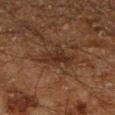Recorded during total-body skin imaging; not selected for excision or biopsy.
A region of skin cropped from a whole-body photographic capture, roughly 15 mm wide.
Imaged with cross-polarized lighting.
The lesion's longest dimension is about 3.5 mm.
Located on the leg.
A male patient, aged around 60.